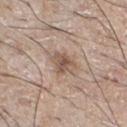Assessment: This lesion was catalogued during total-body skin photography and was not selected for biopsy. Background: A 15 mm crop from a total-body photograph taken for skin-cancer surveillance. Approximately 3 mm at its widest. From the chest. A male subject in their mid- to late 60s. Captured under white-light illumination.